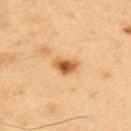<case>
<biopsy_status>not biopsied; imaged during a skin examination</biopsy_status>
<automated_metrics>
  <area_mm2_approx>5.0</area_mm2_approx>
  <eccentricity>0.7</eccentricity>
  <cielab_L>47</cielab_L>
  <cielab_a>20</cielab_a>
  <cielab_b>35</cielab_b>
  <vs_skin_darker_L>12.0</vs_skin_darker_L>
  <vs_skin_contrast_norm>9.5</vs_skin_contrast_norm>
  <border_irregularity_0_10>3.0</border_irregularity_0_10>
  <color_variation_0_10>3.5</color_variation_0_10>
  <peripheral_color_asymmetry>1.0</peripheral_color_asymmetry>
  <nevus_likeness_0_100>95</nevus_likeness_0_100>
  <lesion_detection_confidence_0_100>100</lesion_detection_confidence_0_100>
</automated_metrics>
<patient>
  <sex>male</sex>
  <age_approx>65</age_approx>
</patient>
<site>chest</site>
<lesion_size>
  <long_diameter_mm_approx>3.0</long_diameter_mm_approx>
</lesion_size>
<image>
  <source>total-body photography crop</source>
  <field_of_view_mm>15</field_of_view_mm>
</image>
</case>